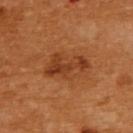No biopsy was performed on this lesion — it was imaged during a full skin examination and was not determined to be concerning.
From the upper back.
A female patient, aged 53–57.
The tile uses cross-polarized illumination.
The lesion's longest dimension is about 5.5 mm.
A 15 mm close-up tile from a total-body photography series done for melanoma screening.
Automated image analysis of the tile measured a lesion area of about 11 mm², a shape eccentricity near 0.85, and a symmetry-axis asymmetry near 0.35. The software also gave a border-irregularity index near 4/10, internal color variation of about 5 on a 0–10 scale, and peripheral color asymmetry of about 1.5. It also reported a detector confidence of about 100 out of 100 that the crop contains a lesion.